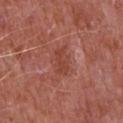Imaged during a routine full-body skin examination; the lesion was not biopsied and no histopathology is available. This image is a 15 mm lesion crop taken from a total-body photograph. The patient is a male aged 63 to 67. Approximately 3.5 mm at its widest. Imaged with white-light lighting. An algorithmic analysis of the crop reported a lesion color around L≈44 a*≈28 b*≈30 in CIELAB, roughly 7 lightness units darker than nearby skin, and a lesion-to-skin contrast of about 5.5 (normalized; higher = more distinct). And it measured a nevus-likeness score of about 0/100. Located on the chest.